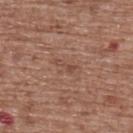- workup — catalogued during a skin exam; not biopsied
- image — total-body-photography crop, ~15 mm field of view
- site — the upper back
- patient — female, roughly 75 years of age